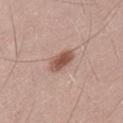| feature | finding |
|---|---|
| workup | no biopsy performed (imaged during a skin exam) |
| automated metrics | a nevus-likeness score of about 95/100 |
| subject | male, in their 50s |
| acquisition | total-body-photography crop, ~15 mm field of view |
| size | about 3.5 mm |
| site | the back |
| illumination | white-light |